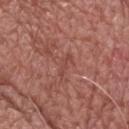{
  "biopsy_status": "not biopsied; imaged during a skin examination",
  "image": {
    "source": "total-body photography crop",
    "field_of_view_mm": 15
  },
  "site": "head or neck",
  "automated_metrics": {
    "area_mm2_approx": 2.5,
    "eccentricity": 0.95,
    "shape_asymmetry": 0.55,
    "border_irregularity_0_10": 7.5,
    "color_variation_0_10": 0.0,
    "peripheral_color_asymmetry": 0.0
  },
  "lighting": "white-light",
  "patient": {
    "sex": "male",
    "age_approx": 70
  }
}